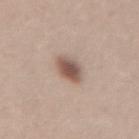<case>
  <biopsy_status>not biopsied; imaged during a skin examination</biopsy_status>
  <image>
    <source>total-body photography crop</source>
    <field_of_view_mm>15</field_of_view_mm>
  </image>
  <patient>
    <sex>female</sex>
    <age_approx>30</age_approx>
  </patient>
  <site>lower back</site>
  <automated_metrics>
    <area_mm2_approx>7.0</area_mm2_approx>
    <eccentricity>0.7</eccentricity>
    <shape_asymmetry>0.1</shape_asymmetry>
    <cielab_L>54</cielab_L>
    <cielab_a>17</cielab_a>
    <cielab_b>24</cielab_b>
    <vs_skin_contrast_norm>9.0</vs_skin_contrast_norm>
  </automated_metrics>
  <lesion_size>
    <long_diameter_mm_approx>3.5</long_diameter_mm_approx>
  </lesion_size>
</case>